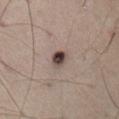Imaged during a routine full-body skin examination; the lesion was not biopsied and no histopathology is available.
A male subject aged 53–57.
Automated image analysis of the tile measured an area of roughly 4 mm², an eccentricity of roughly 0.5, and a symmetry-axis asymmetry near 0.15.
The lesion is on the abdomen.
The tile uses cross-polarized illumination.
A 15 mm close-up tile from a total-body photography series done for melanoma screening.